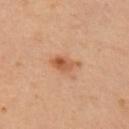– workup · total-body-photography surveillance lesion; no biopsy
– acquisition · ~15 mm crop, total-body skin-cancer survey
– site · the left arm
– subject · female, aged around 40